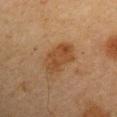Image and clinical context:
Located on the right upper arm. Captured under cross-polarized illumination. A male patient, aged around 65. About 5 mm across. A close-up tile cropped from a whole-body skin photograph, about 15 mm across.